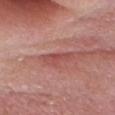Clinical impression: The lesion was photographed on a routine skin check and not biopsied; there is no pathology result. Background: An algorithmic analysis of the crop reported a border-irregularity index near 4.5/10, internal color variation of about 0.5 on a 0–10 scale, and peripheral color asymmetry of about 0. And it measured a detector confidence of about 55 out of 100 that the crop contains a lesion. The lesion's longest dimension is about 3.5 mm. On the head or neck. A close-up tile cropped from a whole-body skin photograph, about 15 mm across. A male subject approximately 60 years of age. The tile uses white-light illumination.